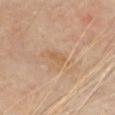follow-up: total-body-photography surveillance lesion; no biopsy | imaging modality: total-body-photography crop, ~15 mm field of view | automated metrics: a peripheral color-asymmetry measure near 0.5; lesion-presence confidence of about 100/100 | location: the chest | patient: female, aged 58–62.